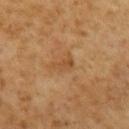Notes:
• follow-up: total-body-photography surveillance lesion; no biopsy
• automated metrics: a lesion area of about 4 mm² and a shape-asymmetry score of about 0.5 (0 = symmetric); a lesion–skin lightness drop of about 6 and a normalized border contrast of about 5.5; a classifier nevus-likeness of about 0/100 and a lesion-detection confidence of about 100/100
• illumination: cross-polarized illumination
• subject: male, approximately 60 years of age
• location: the mid back
• acquisition: ~15 mm crop, total-body skin-cancer survey
• size: about 3 mm